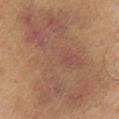{"biopsy_status": "not biopsied; imaged during a skin examination", "site": "chest", "patient": {"sex": "male", "age_approx": 65}, "image": {"source": "total-body photography crop", "field_of_view_mm": 15}}